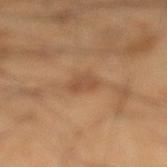Q: Was this lesion biopsied?
A: no biopsy performed (imaged during a skin exam)
Q: What did automated image analysis measure?
A: an area of roughly 5 mm², a shape eccentricity near 0.6, and two-axis asymmetry of about 0.2; a mean CIELAB color near L≈50 a*≈20 b*≈34, roughly 8 lightness units darker than nearby skin, and a normalized border contrast of about 5.5; border irregularity of about 2 on a 0–10 scale and internal color variation of about 2.5 on a 0–10 scale
Q: Patient demographics?
A: male, about 40 years old
Q: Where on the body is the lesion?
A: the left lower leg
Q: What kind of image is this?
A: total-body-photography crop, ~15 mm field of view
Q: What is the lesion's diameter?
A: ~3 mm (longest diameter)
Q: How was the tile lit?
A: cross-polarized illumination